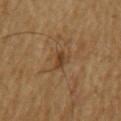notes: no biopsy performed (imaged during a skin exam); lesion size: ~2.5 mm (longest diameter); subject: male, in their mid-60s; acquisition: ~15 mm tile from a whole-body skin photo; location: the arm.